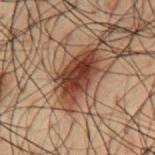Q: Was a biopsy performed?
A: no biopsy performed (imaged during a skin exam)
Q: What kind of image is this?
A: total-body-photography crop, ~15 mm field of view
Q: What are the patient's age and sex?
A: male, about 50 years old
Q: What did automated image analysis measure?
A: an average lesion color of about L≈30 a*≈17 b*≈23 (CIELAB); a within-lesion color-variation index near 6/10 and peripheral color asymmetry of about 2; an automated nevus-likeness rating near 95 out of 100 and lesion-presence confidence of about 100/100
Q: How was the tile lit?
A: cross-polarized illumination
Q: How large is the lesion?
A: ≈6.5 mm
Q: Where on the body is the lesion?
A: the mid back